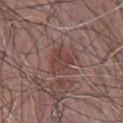* biopsy status: catalogued during a skin exam; not biopsied
* patient: male, in their mid- to late 60s
* acquisition: total-body-photography crop, ~15 mm field of view
* body site: the chest
* automated lesion analysis: a lesion area of about 8.5 mm², an eccentricity of roughly 0.5, and two-axis asymmetry of about 0.25; a border-irregularity index near 3/10, a within-lesion color-variation index near 4/10, and radial color variation of about 1.5